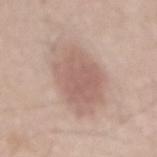Captured during whole-body skin photography for melanoma surveillance; the lesion was not biopsied. The tile uses white-light illumination. A male subject, aged approximately 45. The lesion is located on the mid back. Longest diameter approximately 8 mm. A 15 mm crop from a total-body photograph taken for skin-cancer surveillance.